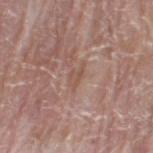Impression: Recorded during total-body skin imaging; not selected for excision or biopsy. Acquisition and patient details: This is a white-light tile. On the right thigh. Automated image analysis of the tile measured an eccentricity of roughly 0.9 and a symmetry-axis asymmetry near 0.45. The analysis additionally found border irregularity of about 5 on a 0–10 scale. And it measured a classifier nevus-likeness of about 0/100. Approximately 3 mm at its widest. This image is a 15 mm lesion crop taken from a total-body photograph. The subject is a female aged 58–62.